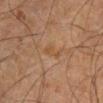Notes:
• follow-up — imaged on a skin check; not biopsied
• body site — the abdomen
• tile lighting — cross-polarized illumination
• subject — male, roughly 80 years of age
• image source — ~15 mm crop, total-body skin-cancer survey
• automated metrics — an eccentricity of roughly 0.9 and a symmetry-axis asymmetry near 0.4; an automated nevus-likeness rating near 0 out of 100
• size — ≈3 mm